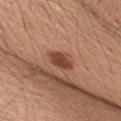Captured during whole-body skin photography for melanoma surveillance; the lesion was not biopsied. A male subject, aged 28 to 32. Located on the left upper arm. A 15 mm close-up tile from a total-body photography series done for melanoma screening. About 3.5 mm across. Imaged with white-light lighting.